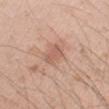| field | value |
|---|---|
| notes | no biopsy performed (imaged during a skin exam) |
| location | the right forearm |
| patient | male, roughly 25 years of age |
| acquisition | ~15 mm crop, total-body skin-cancer survey |
| automated metrics | an area of roughly 4 mm², a shape eccentricity near 0.75, and two-axis asymmetry of about 0.35; radial color variation of about 1; an automated nevus-likeness rating near 5 out of 100 and a detector confidence of about 100 out of 100 that the crop contains a lesion |
| lesion size | ≈2.5 mm |